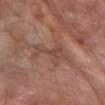lesion_size:
  long_diameter_mm_approx: 4.5
patient:
  sex: female
  age_approx: 70
lighting: white-light
site: left forearm
image:
  source: total-body photography crop
  field_of_view_mm: 15